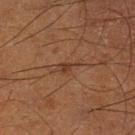Q: What did automated image analysis measure?
A: internal color variation of about 1.5 on a 0–10 scale and peripheral color asymmetry of about 0.5
Q: Lesion location?
A: the left lower leg
Q: How large is the lesion?
A: ≈3 mm
Q: What are the patient's age and sex?
A: male, aged 63 to 67
Q: How was this image acquired?
A: 15 mm crop, total-body photography
Q: How was the tile lit?
A: cross-polarized